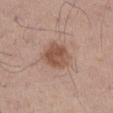Imaged during a routine full-body skin examination; the lesion was not biopsied and no histopathology is available. This is a white-light tile. A male patient, about 55 years old. A region of skin cropped from a whole-body photographic capture, roughly 15 mm wide. From the abdomen. The lesion-visualizer software estimated a border-irregularity rating of about 2/10, internal color variation of about 3.5 on a 0–10 scale, and a peripheral color-asymmetry measure near 1.5. It also reported an automated nevus-likeness rating near 30 out of 100 and lesion-presence confidence of about 100/100.